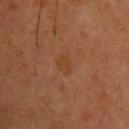Findings:
• workup: no biopsy performed (imaged during a skin exam)
• patient: male, approximately 35 years of age
• lighting: cross-polarized
• lesion diameter: ≈2.5 mm
• location: the upper back
• acquisition: ~15 mm tile from a whole-body skin photo
• automated metrics: a lesion–skin lightness drop of about 3 and a lesion-to-skin contrast of about 5 (normalized; higher = more distinct); a border-irregularity rating of about 2.5/10 and radial color variation of about 0.5; a classifier nevus-likeness of about 15/100 and a detector confidence of about 100 out of 100 that the crop contains a lesion